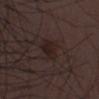Q: Is there a histopathology result?
A: imaged on a skin check; not biopsied
Q: Who is the patient?
A: male, aged around 50
Q: Illumination type?
A: white-light
Q: What is the lesion's diameter?
A: ~4 mm (longest diameter)
Q: How was this image acquired?
A: ~15 mm crop, total-body skin-cancer survey